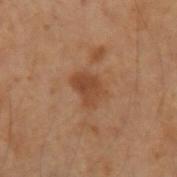Clinical impression: Part of a total-body skin-imaging series; this lesion was reviewed on a skin check and was not flagged for biopsy.